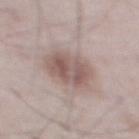Q: What are the patient's age and sex?
A: male, aged 53–57
Q: Lesion location?
A: the abdomen
Q: How was this image acquired?
A: ~15 mm crop, total-body skin-cancer survey